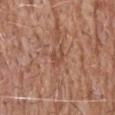No biopsy was performed on this lesion — it was imaged during a full skin examination and was not determined to be concerning.